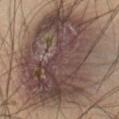Captured during whole-body skin photography for melanoma surveillance; the lesion was not biopsied. The lesion is on the abdomen. This is a white-light tile. A close-up tile cropped from a whole-body skin photograph, about 15 mm across. The lesion's longest dimension is about 13.5 mm. A male subject aged 53–57.